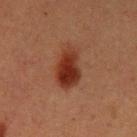Q: Was a biopsy performed?
A: catalogued during a skin exam; not biopsied
Q: What did automated image analysis measure?
A: a lesion color around L≈27 a*≈23 b*≈27 in CIELAB, roughly 11 lightness units darker than nearby skin, and a normalized border contrast of about 11; a within-lesion color-variation index near 4.5/10 and a peripheral color-asymmetry measure near 1.5; a nevus-likeness score of about 100/100 and lesion-presence confidence of about 100/100
Q: Where on the body is the lesion?
A: the left upper arm
Q: Illumination type?
A: cross-polarized illumination
Q: What kind of image is this?
A: ~15 mm crop, total-body skin-cancer survey
Q: What are the patient's age and sex?
A: male, aged approximately 40
Q: Lesion size?
A: ~4.5 mm (longest diameter)